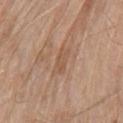{"biopsy_status": "not biopsied; imaged during a skin examination", "image": {"source": "total-body photography crop", "field_of_view_mm": 15}, "automated_metrics": {"area_mm2_approx": 4.5, "eccentricity": 0.9, "shape_asymmetry": 0.3, "vs_skin_darker_L": 7.0, "vs_skin_contrast_norm": 5.5, "border_irregularity_0_10": 3.5, "color_variation_0_10": 2.0, "nevus_likeness_0_100": 0, "lesion_detection_confidence_0_100": 85}, "lighting": "white-light", "patient": {"sex": "male", "age_approx": 80}, "site": "chest"}